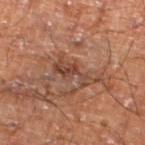Clinical impression:
Imaged during a routine full-body skin examination; the lesion was not biopsied and no histopathology is available.
Acquisition and patient details:
Captured under cross-polarized illumination. Located on the left lower leg. A male subject, in their 60s. The lesion's longest dimension is about 5 mm. A region of skin cropped from a whole-body photographic capture, roughly 15 mm wide.